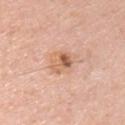Background:
A male subject in their 50s. This is a white-light tile. A 15 mm close-up extracted from a 3D total-body photography capture. From the chest.